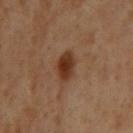<lesion>
<biopsy_status>not biopsied; imaged during a skin examination</biopsy_status>
<patient>
  <sex>male</sex>
  <age_approx>60</age_approx>
</patient>
<lighting>cross-polarized</lighting>
<automated_metrics>
  <area_mm2_approx>6.5</area_mm2_approx>
  <eccentricity>0.65</eccentricity>
  <shape_asymmetry>0.2</shape_asymmetry>
</automated_metrics>
<site>mid back</site>
<image>
  <source>total-body photography crop</source>
  <field_of_view_mm>15</field_of_view_mm>
</image>
</lesion>